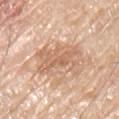workup = imaged on a skin check; not biopsied | location = the left arm | illumination = white-light illumination | patient = male, aged around 80 | image = ~15 mm tile from a whole-body skin photo.